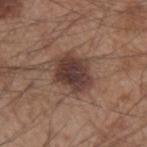Impression:
Part of a total-body skin-imaging series; this lesion was reviewed on a skin check and was not flagged for biopsy.
Image and clinical context:
On the left forearm. The patient is a male aged 53–57. A region of skin cropped from a whole-body photographic capture, roughly 15 mm wide.